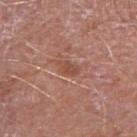The tile uses white-light illumination. The patient is a male aged 68 to 72. About 3 mm across. On the right upper arm. Cropped from a whole-body photographic skin survey; the tile spans about 15 mm.